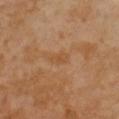Q: Is there a histopathology result?
A: total-body-photography surveillance lesion; no biopsy
Q: What kind of image is this?
A: ~15 mm crop, total-body skin-cancer survey
Q: What is the anatomic site?
A: the chest
Q: What are the patient's age and sex?
A: female, in their mid-50s